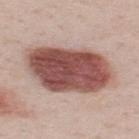Captured during whole-body skin photography for melanoma surveillance; the lesion was not biopsied. From the upper back. Captured under white-light illumination. An algorithmic analysis of the crop reported a nevus-likeness score of about 100/100 and lesion-presence confidence of about 100/100. About 10 mm across. A male patient, about 50 years old. Cropped from a total-body skin-imaging series; the visible field is about 15 mm.